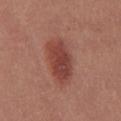| field | value |
|---|---|
| notes | no biopsy performed (imaged during a skin exam) |
| patient | female, aged 23 to 27 |
| size | about 6.5 mm |
| lighting | white-light illumination |
| acquisition | total-body-photography crop, ~15 mm field of view |
| site | the chest |